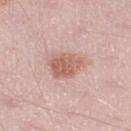Q: Was a biopsy performed?
A: catalogued during a skin exam; not biopsied
Q: How was the tile lit?
A: white-light
Q: What did automated image analysis measure?
A: a border-irregularity rating of about 2/10, a within-lesion color-variation index near 3.5/10, and radial color variation of about 1.5
Q: Patient demographics?
A: male, in their 50s
Q: What kind of image is this?
A: ~15 mm tile from a whole-body skin photo
Q: What is the anatomic site?
A: the right lower leg
Q: Lesion size?
A: ≈4 mm